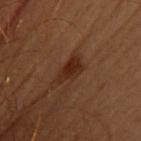follow-up = total-body-photography surveillance lesion; no biopsy
anatomic site = the back
diameter = about 4 mm
patient = female, aged 48 to 52
image = ~15 mm tile from a whole-body skin photo
tile lighting = cross-polarized